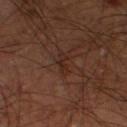workup = imaged on a skin check; not biopsied
site = the right thigh
tile lighting = cross-polarized illumination
subject = male, in their 60s
size = ~2.5 mm (longest diameter)
image = ~15 mm crop, total-body skin-cancer survey